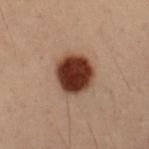follow-up: no biopsy performed (imaged during a skin exam) | lesion size: ~4 mm (longest diameter) | lighting: cross-polarized | patient: male, in their mid- to late 50s | imaging modality: ~15 mm tile from a whole-body skin photo | automated metrics: an area of roughly 14 mm², an eccentricity of roughly 0.35, and two-axis asymmetry of about 0.1; a mean CIELAB color near L≈28 a*≈19 b*≈23, roughly 19 lightness units darker than nearby skin, and a lesion-to-skin contrast of about 16.5 (normalized; higher = more distinct); a border-irregularity rating of about 1/10, a within-lesion color-variation index near 4/10, and peripheral color asymmetry of about 1; a nevus-likeness score of about 100/100 and a lesion-detection confidence of about 100/100 | location: the left forearm.